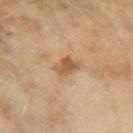location — the left forearm
image — 15 mm crop, total-body photography
patient — female, aged 58–62
lighting — cross-polarized
lesion size — ~3 mm (longest diameter)